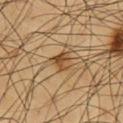A close-up tile cropped from a whole-body skin photograph, about 15 mm across.
The subject is a male roughly 60 years of age.
On the chest.
Approximately 3 mm at its widest.
The total-body-photography lesion software estimated a footprint of about 5 mm², a shape eccentricity near 0.45, and a symmetry-axis asymmetry near 0.35. And it measured a classifier nevus-likeness of about 80/100 and lesion-presence confidence of about 100/100.